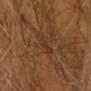biopsy status: imaged on a skin check; not biopsied | subject: male, aged around 65 | anatomic site: the head or neck | image-analysis metrics: a footprint of about 3.5 mm²; a lesion–skin lightness drop of about 5 and a normalized border contrast of about 5; a classifier nevus-likeness of about 0/100 | image: 15 mm crop, total-body photography | lighting: cross-polarized illumination | diameter: about 3 mm.